The lesion is located on the upper back · a region of skin cropped from a whole-body photographic capture, roughly 15 mm wide · the patient is a female aged around 40 · captured under cross-polarized illumination.
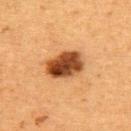Histopathologically confirmed as a dysplastic (Clark) nevus — a benign skin lesion.